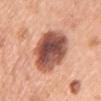Clinical impression:
The lesion was tiled from a total-body skin photograph and was not biopsied.
Clinical summary:
A 15 mm close-up extracted from a 3D total-body photography capture. Captured under white-light illumination. The recorded lesion diameter is about 6.5 mm. Located on the left upper arm. A female subject in their 60s.